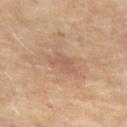Assessment:
Recorded during total-body skin imaging; not selected for excision or biopsy.
Context:
A region of skin cropped from a whole-body photographic capture, roughly 15 mm wide. The lesion is on the left thigh. Approximately 3 mm at its widest. The lesion-visualizer software estimated a border-irregularity index near 6.5/10 and radial color variation of about 0. The software also gave a nevus-likeness score of about 0/100 and a lesion-detection confidence of about 100/100. The patient is a female aged 48–52. The tile uses cross-polarized illumination.